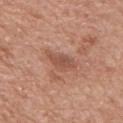{"patient": {"sex": "male", "age_approx": 80}, "lighting": "white-light", "image": {"source": "total-body photography crop", "field_of_view_mm": 15}, "site": "upper back", "lesion_size": {"long_diameter_mm_approx": 4.0}, "automated_metrics": {"area_mm2_approx": 6.0, "shape_asymmetry": 0.3, "cielab_L": 52, "cielab_a": 23, "cielab_b": 29, "vs_skin_contrast_norm": 6.0, "border_irregularity_0_10": 3.5, "color_variation_0_10": 1.5, "peripheral_color_asymmetry": 0.5, "nevus_likeness_0_100": 0, "lesion_detection_confidence_0_100": 100}}